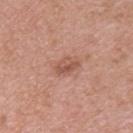This lesion was catalogued during total-body skin photography and was not selected for biopsy. A female subject approximately 40 years of age. About 3 mm across. A roughly 15 mm field-of-view crop from a total-body skin photograph. The lesion is located on the back. This is a white-light tile.